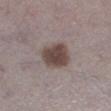{
  "biopsy_status": "not biopsied; imaged during a skin examination",
  "image": {
    "source": "total-body photography crop",
    "field_of_view_mm": 15
  },
  "site": "left lower leg",
  "lighting": "white-light",
  "patient": {
    "sex": "male",
    "age_approx": 70
  }
}